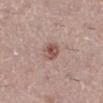Captured under white-light illumination. The patient is a female aged 38–42. From the right lower leg. A lesion tile, about 15 mm wide, cut from a 3D total-body photograph. The lesion-visualizer software estimated a footprint of about 4.5 mm², an outline eccentricity of about 0.45 (0 = round, 1 = elongated), and two-axis asymmetry of about 0.2. The analysis additionally found a mean CIELAB color near L≈52 a*≈20 b*≈23 and roughly 12 lightness units darker than nearby skin. And it measured a border-irregularity rating of about 1.5/10, internal color variation of about 4 on a 0–10 scale, and a peripheral color-asymmetry measure near 1.5. Measured at roughly 2.5 mm in maximum diameter.